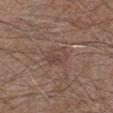subject: male, in their 60s | illumination: white-light illumination | size: about 3.5 mm | image-analysis metrics: a lesion–skin lightness drop of about 6 and a normalized lesion–skin contrast near 5 | site: the left lower leg | image source: 15 mm crop, total-body photography.